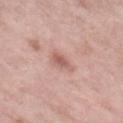No biopsy was performed on this lesion — it was imaged during a full skin examination and was not determined to be concerning. On the right lower leg. A female subject, aged 68–72. Longest diameter approximately 3 mm. A 15 mm close-up tile from a total-body photography series done for melanoma screening.